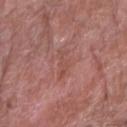| field | value |
|---|---|
| follow-up | imaged on a skin check; not biopsied |
| image-analysis metrics | a lesion area of about 4 mm², an outline eccentricity of about 0.95 (0 = round, 1 = elongated), and a symmetry-axis asymmetry near 0.45; a lesion color around L≈50 a*≈24 b*≈25 in CIELAB and a lesion–skin lightness drop of about 6 |
| patient | male, roughly 80 years of age |
| imaging modality | 15 mm crop, total-body photography |
| location | the arm |
| lesion diameter | ≈3.5 mm |
| lighting | white-light illumination |